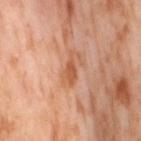The lesion was photographed on a routine skin check and not biopsied; there is no pathology result. The lesion is located on the right thigh. A region of skin cropped from a whole-body photographic capture, roughly 15 mm wide. A female subject, aged 53–57. Longest diameter approximately 3.5 mm.